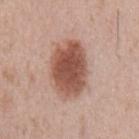notes: catalogued during a skin exam; not biopsied | image: 15 mm crop, total-body photography | subject: male, aged approximately 65 | anatomic site: the chest | lighting: white-light.